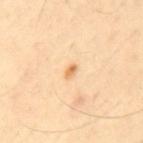image source: ~15 mm crop, total-body skin-cancer survey; location: the back; patient: male, aged approximately 55.